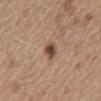Case summary:
* acquisition: ~15 mm tile from a whole-body skin photo
* patient: male, aged 68 to 72
* TBP lesion metrics: an area of roughly 4 mm² and two-axis asymmetry of about 0.25; a mean CIELAB color near L≈47 a*≈18 b*≈28 and a lesion-to-skin contrast of about 10 (normalized; higher = more distinct); a border-irregularity index near 2/10, a within-lesion color-variation index near 6/10, and peripheral color asymmetry of about 1.5; a classifier nevus-likeness of about 90/100 and lesion-presence confidence of about 100/100
* location: the back
* tile lighting: white-light illumination
* size: ~2.5 mm (longest diameter)